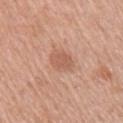biopsy_status: not biopsied; imaged during a skin examination
image:
  source: total-body photography crop
  field_of_view_mm: 15
patient:
  sex: female
  age_approx: 45
site: arm